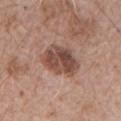{
  "biopsy_status": "not biopsied; imaged during a skin examination",
  "patient": {
    "sex": "male",
    "age_approx": 65
  },
  "site": "chest",
  "image": {
    "source": "total-body photography crop",
    "field_of_view_mm": 15
  }
}